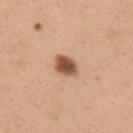follow-up: imaged on a skin check; not biopsied
imaging modality: total-body-photography crop, ~15 mm field of view
subject: female, approximately 30 years of age
location: the right upper arm
automated metrics: a lesion color around L≈54 a*≈22 b*≈31 in CIELAB, a lesion–skin lightness drop of about 17, and a lesion-to-skin contrast of about 11 (normalized; higher = more distinct); a lesion-detection confidence of about 100/100
lesion size: ~3 mm (longest diameter)